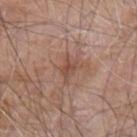* follow-up · catalogued during a skin exam; not biopsied
* acquisition · 15 mm crop, total-body photography
* body site · the left arm
* subject · male, aged approximately 80